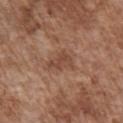notes: total-body-photography surveillance lesion; no biopsy
subject: male, aged 73 to 77
location: the chest
TBP lesion metrics: a footprint of about 5.5 mm², an eccentricity of roughly 0.75, and a symmetry-axis asymmetry near 0.4; peripheral color asymmetry of about 1; an automated nevus-likeness rating near 0 out of 100 and lesion-presence confidence of about 100/100
size: ~3.5 mm (longest diameter)
image: ~15 mm crop, total-body skin-cancer survey
lighting: white-light illumination